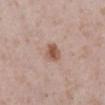No biopsy was performed on this lesion — it was imaged during a full skin examination and was not determined to be concerning.
A 15 mm close-up tile from a total-body photography series done for melanoma screening.
The lesion is on the leg.
A female subject aged 28–32.
Imaged with white-light lighting.